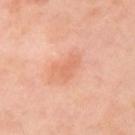Imaged during a routine full-body skin examination; the lesion was not biopsied and no histopathology is available. About 4 mm across. The subject is a female in their mid-50s. The lesion is located on the left upper arm. The tile uses cross-polarized illumination. A lesion tile, about 15 mm wide, cut from a 3D total-body photograph.